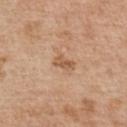Captured during whole-body skin photography for melanoma surveillance; the lesion was not biopsied. A male patient, roughly 55 years of age. This is a white-light tile. Cropped from a whole-body photographic skin survey; the tile spans about 15 mm. Approximately 2.5 mm at its widest. From the chest.